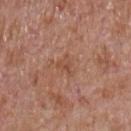Recorded during total-body skin imaging; not selected for excision or biopsy. Located on the chest. Cropped from a whole-body photographic skin survey; the tile spans about 15 mm. A male subject approximately 65 years of age.